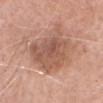Clinical impression: Captured during whole-body skin photography for melanoma surveillance; the lesion was not biopsied. Background: The lesion is on the head or neck. A female patient approximately 75 years of age. The recorded lesion diameter is about 6 mm. The tile uses white-light illumination. A lesion tile, about 15 mm wide, cut from a 3D total-body photograph.